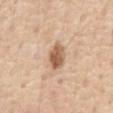Case summary:
* workup — no biopsy performed (imaged during a skin exam)
* image source — total-body-photography crop, ~15 mm field of view
* size — ≈4 mm
* subject — male, in their mid- to late 70s
* site — the abdomen
* lighting — white-light